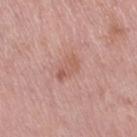Assessment: The lesion was tiled from a total-body skin photograph and was not biopsied. Background: Located on the leg. A female patient, aged around 40. A 15 mm close-up extracted from a 3D total-body photography capture.